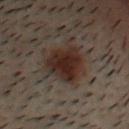biopsy_status: not biopsied; imaged during a skin examination
image:
  source: total-body photography crop
  field_of_view_mm: 15
site: head or neck
lighting: cross-polarized
patient:
  sex: male
  age_approx: 30
lesion_size:
  long_diameter_mm_approx: 5.0